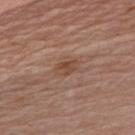Clinical impression: This lesion was catalogued during total-body skin photography and was not selected for biopsy. Clinical summary: The recorded lesion diameter is about 2.5 mm. A lesion tile, about 15 mm wide, cut from a 3D total-body photograph. Captured under white-light illumination. From the front of the torso. A male subject aged around 70.